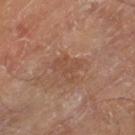Captured during whole-body skin photography for melanoma surveillance; the lesion was not biopsied. An algorithmic analysis of the crop reported an area of roughly 6.5 mm², an outline eccentricity of about 0.65 (0 = round, 1 = elongated), and a symmetry-axis asymmetry near 0.45. The analysis additionally found an average lesion color of about L≈46 a*≈20 b*≈29 (CIELAB), about 6 CIELAB-L* units darker than the surrounding skin, and a normalized lesion–skin contrast near 4.5. The analysis additionally found a color-variation rating of about 2/10 and radial color variation of about 0.5. From the leg. The subject is a male aged around 70. A 15 mm close-up tile from a total-body photography series done for melanoma screening.